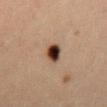No biopsy was performed on this lesion — it was imaged during a full skin examination and was not determined to be concerning.
The lesion is on the abdomen.
This image is a 15 mm lesion crop taken from a total-body photograph.
Approximately 2.5 mm at its widest.
The subject is a female aged approximately 40.
The tile uses cross-polarized illumination.